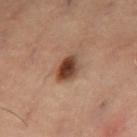No biopsy was performed on this lesion — it was imaged during a full skin examination and was not determined to be concerning. Located on the abdomen. A roughly 15 mm field-of-view crop from a total-body skin photograph. The patient is a female aged approximately 55.